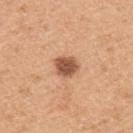{
  "biopsy_status": "not biopsied; imaged during a skin examination",
  "image": {
    "source": "total-body photography crop",
    "field_of_view_mm": 15
  },
  "patient": {
    "sex": "male",
    "age_approx": 50
  },
  "site": "upper back"
}